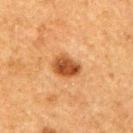Clinical impression:
The lesion was photographed on a routine skin check and not biopsied; there is no pathology result.
Context:
Imaged with cross-polarized lighting. A male subject in their mid-70s. The lesion is on the right upper arm. A 15 mm close-up extracted from a 3D total-body photography capture.